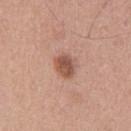Imaged during a routine full-body skin examination; the lesion was not biopsied and no histopathology is available. Located on the chest. A lesion tile, about 15 mm wide, cut from a 3D total-body photograph. About 3 mm across. A male patient, approximately 55 years of age. The tile uses white-light illumination.